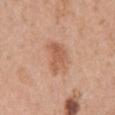Recorded during total-body skin imaging; not selected for excision or biopsy. Imaged with white-light lighting. The recorded lesion diameter is about 4.5 mm. A female patient in their mid- to late 50s. A region of skin cropped from a whole-body photographic capture, roughly 15 mm wide. The lesion is on the left upper arm.